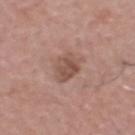| feature | finding |
|---|---|
| follow-up | imaged on a skin check; not biopsied |